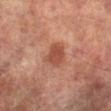The lesion was photographed on a routine skin check and not biopsied; there is no pathology result. Approximately 3 mm at its widest. Located on the left lower leg. A 15 mm crop from a total-body photograph taken for skin-cancer surveillance. The patient is a male approximately 75 years of age. Imaged with cross-polarized lighting.